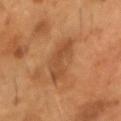follow-up: catalogued during a skin exam; not biopsied | automated lesion analysis: a border-irregularity rating of about 4.5/10 and internal color variation of about 2.5 on a 0–10 scale; lesion-presence confidence of about 100/100 | body site: the head or neck | tile lighting: cross-polarized illumination | imaging modality: total-body-photography crop, ~15 mm field of view | patient: male, about 55 years old | lesion size: ~5 mm (longest diameter).